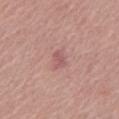Part of a total-body skin-imaging series; this lesion was reviewed on a skin check and was not flagged for biopsy. A male subject in their 60s. Automated tile analysis of the lesion measured a footprint of about 3 mm², an outline eccentricity of about 0.8 (0 = round, 1 = elongated), and a shape-asymmetry score of about 0.45 (0 = symmetric). The software also gave a mean CIELAB color near L≈55 a*≈25 b*≈20, roughly 8 lightness units darker than nearby skin, and a lesion-to-skin contrast of about 5.5 (normalized; higher = more distinct). The analysis additionally found border irregularity of about 4.5 on a 0–10 scale and internal color variation of about 1 on a 0–10 scale. Cropped from a whole-body photographic skin survey; the tile spans about 15 mm. Measured at roughly 2.5 mm in maximum diameter. Imaged with white-light lighting. From the front of the torso.